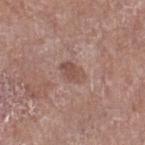Image and clinical context:
Located on the right thigh. The patient is a female aged around 75. About 3 mm across. The total-body-photography lesion software estimated a lesion color around L≈51 a*≈19 b*≈24 in CIELAB, about 8 CIELAB-L* units darker than the surrounding skin, and a normalized lesion–skin contrast near 6.5. The software also gave a border-irregularity rating of about 2/10 and internal color variation of about 3 on a 0–10 scale. Captured under white-light illumination. A close-up tile cropped from a whole-body skin photograph, about 15 mm across.